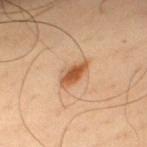Imaged during a routine full-body skin examination; the lesion was not biopsied and no histopathology is available. The patient is a male in their mid-40s. About 4 mm across. An algorithmic analysis of the crop reported a nevus-likeness score of about 95/100 and a lesion-detection confidence of about 100/100. This is a cross-polarized tile. The lesion is on the upper back. A lesion tile, about 15 mm wide, cut from a 3D total-body photograph.